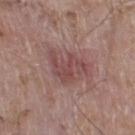biopsy_status: not biopsied; imaged during a skin examination
lighting: white-light
patient:
  sex: male
  age_approx: 60
site: left thigh
image:
  source: total-body photography crop
  field_of_view_mm: 15